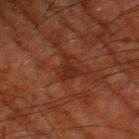Clinical impression:
Imaged during a routine full-body skin examination; the lesion was not biopsied and no histopathology is available.
Image and clinical context:
An algorithmic analysis of the crop reported a lesion color around L≈21 a*≈20 b*≈24 in CIELAB, about 5 CIELAB-L* units darker than the surrounding skin, and a normalized border contrast of about 6.5. The software also gave a border-irregularity rating of about 6/10 and internal color variation of about 2 on a 0–10 scale. The lesion is located on the right thigh. Cropped from a total-body skin-imaging series; the visible field is about 15 mm. A male patient, roughly 80 years of age. Measured at roughly 3.5 mm in maximum diameter.